Cropped from a total-body skin-imaging series; the visible field is about 15 mm · the lesion's longest dimension is about 4.5 mm · Automated image analysis of the tile measured an area of roughly 7 mm², a shape eccentricity near 0.85, and two-axis asymmetry of about 0.25. It also reported a lesion color around L≈43 a*≈22 b*≈25 in CIELAB, about 13 CIELAB-L* units darker than the surrounding skin, and a normalized lesion–skin contrast near 10.5 · the subject is a female about 50 years old · this is a white-light tile · from the upper back: 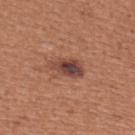The biopsy diagnosis was a dysplastic (Clark) nevus, classified as a benign skin lesion.